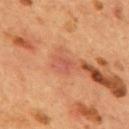Q: Was this lesion biopsied?
A: total-body-photography surveillance lesion; no biopsy
Q: Where on the body is the lesion?
A: the back
Q: How was the tile lit?
A: cross-polarized illumination
Q: What is the imaging modality?
A: ~15 mm tile from a whole-body skin photo
Q: What did automated image analysis measure?
A: a footprint of about 3 mm², an outline eccentricity of about 0.7 (0 = round, 1 = elongated), and a shape-asymmetry score of about 0.55 (0 = symmetric)
Q: Lesion size?
A: ≈2.5 mm
Q: What are the patient's age and sex?
A: male, in their mid-50s